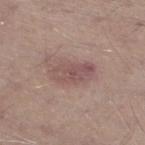Q: Was a biopsy performed?
A: no biopsy performed (imaged during a skin exam)
Q: How large is the lesion?
A: ~5 mm (longest diameter)
Q: Automated lesion metrics?
A: a footprint of about 10 mm², an outline eccentricity of about 0.85 (0 = round, 1 = elongated), and a symmetry-axis asymmetry near 0.2; a border-irregularity rating of about 2.5/10; a nevus-likeness score of about 10/100 and a detector confidence of about 100 out of 100 that the crop contains a lesion
Q: What is the imaging modality?
A: 15 mm crop, total-body photography
Q: What lighting was used for the tile?
A: white-light illumination
Q: Lesion location?
A: the left lower leg
Q: Patient demographics?
A: male, aged around 65